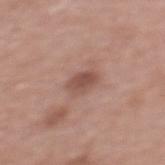Impression: The lesion was photographed on a routine skin check and not biopsied; there is no pathology result. Image and clinical context: Located on the upper back. This image is a 15 mm lesion crop taken from a total-body photograph. A female subject aged 68–72. The total-body-photography lesion software estimated an average lesion color of about L≈50 a*≈21 b*≈25 (CIELAB). The tile uses white-light illumination. Approximately 2.5 mm at its widest.